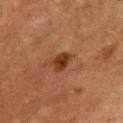<lesion>
<image>
  <source>total-body photography crop</source>
  <field_of_view_mm>15</field_of_view_mm>
</image>
<lighting>cross-polarized</lighting>
<lesion_size>
  <long_diameter_mm_approx>3.0</long_diameter_mm_approx>
</lesion_size>
<patient>
  <sex>male</sex>
  <age_approx>75</age_approx>
</patient>
<site>chest</site>
<automated_metrics>
  <cielab_L>29</cielab_L>
  <cielab_a>21</cielab_a>
  <cielab_b>28</cielab_b>
  <vs_skin_darker_L>10.0</vs_skin_darker_L>
  <vs_skin_contrast_norm>10.0</vs_skin_contrast_norm>
</automated_metrics>
</lesion>